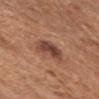Captured during whole-body skin photography for melanoma surveillance; the lesion was not biopsied. Cropped from a total-body skin-imaging series; the visible field is about 15 mm. The lesion is on the head or neck. A female subject, about 75 years old. About 3 mm across.